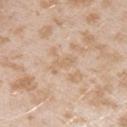Clinical impression: Imaged during a routine full-body skin examination; the lesion was not biopsied and no histopathology is available. Acquisition and patient details: Imaged with white-light lighting. This image is a 15 mm lesion crop taken from a total-body photograph. A female subject, about 25 years old. The lesion is on the left upper arm.